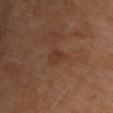| feature | finding |
|---|---|
| biopsy status | imaged on a skin check; not biopsied |
| lesion size | ≈2.5 mm |
| patient | male, aged approximately 70 |
| imaging modality | total-body-photography crop, ~15 mm field of view |
| site | the chest |
| illumination | cross-polarized illumination |